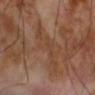- biopsy status: no biopsy performed (imaged during a skin exam)
- subject: male, aged approximately 70
- body site: the arm
- imaging modality: 15 mm crop, total-body photography